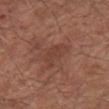workup = no biopsy performed (imaged during a skin exam)
location = the right upper arm
lesion size = ≈3.5 mm
patient = male, in their mid- to late 50s
illumination = white-light illumination
image = 15 mm crop, total-body photography